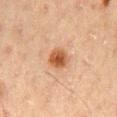{
  "biopsy_status": "not biopsied; imaged during a skin examination",
  "site": "mid back",
  "image": {
    "source": "total-body photography crop",
    "field_of_view_mm": 15
  },
  "patient": {
    "sex": "male",
    "age_approx": 50
  }
}